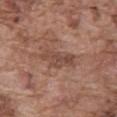<lesion>
  <biopsy_status>not biopsied; imaged during a skin examination</biopsy_status>
  <image>
    <source>total-body photography crop</source>
    <field_of_view_mm>15</field_of_view_mm>
  </image>
  <lesion_size>
    <long_diameter_mm_approx>4.5</long_diameter_mm_approx>
  </lesion_size>
  <lighting>white-light</lighting>
  <patient>
    <sex>male</sex>
    <age_approx>75</age_approx>
  </patient>
  <automated_metrics>
    <eccentricity>0.9</eccentricity>
    <shape_asymmetry>0.4</shape_asymmetry>
    <vs_skin_darker_L>8.0</vs_skin_darker_L>
    <vs_skin_contrast_norm>6.5</vs_skin_contrast_norm>
    <border_irregularity_0_10>5.0</border_irregularity_0_10>
    <color_variation_0_10>3.0</color_variation_0_10>
    <peripheral_color_asymmetry>1.0</peripheral_color_asymmetry>
    <nevus_likeness_0_100>0</nevus_likeness_0_100>
  </automated_metrics>
  <site>abdomen</site>
</lesion>